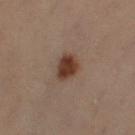Clinical impression: Recorded during total-body skin imaging; not selected for excision or biopsy. Acquisition and patient details: Captured under cross-polarized illumination. Located on the right lower leg. A close-up tile cropped from a whole-body skin photograph, about 15 mm across. Approximately 3 mm at its widest. The total-body-photography lesion software estimated an eccentricity of roughly 0.6 and a shape-asymmetry score of about 0.15 (0 = symmetric). It also reported a detector confidence of about 100 out of 100 that the crop contains a lesion. A female subject aged around 50.